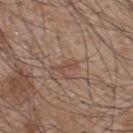  biopsy_status: not biopsied; imaged during a skin examination
  patient:
    sex: male
    age_approx: 45
  automated_metrics:
    area_mm2_approx: 3.0
    shape_asymmetry: 0.4
    border_irregularity_0_10: 4.0
  image:
    source: total-body photography crop
    field_of_view_mm: 15
  lesion_size:
    long_diameter_mm_approx: 3.0
  site: upper back